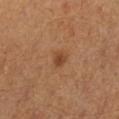follow-up: total-body-photography surveillance lesion; no biopsy | patient: male, about 65 years old | acquisition: 15 mm crop, total-body photography | TBP lesion metrics: a mean CIELAB color near L≈41 a*≈21 b*≈32 and a normalized border contrast of about 7; a border-irregularity index near 2.5/10, a color-variation rating of about 1.5/10, and peripheral color asymmetry of about 0.5; a classifier nevus-likeness of about 80/100 | tile lighting: cross-polarized | location: the left lower leg | diameter: about 2 mm.